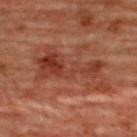Clinical impression: This lesion was catalogued during total-body skin photography and was not selected for biopsy.